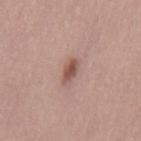Imaged during a routine full-body skin examination; the lesion was not biopsied and no histopathology is available.
A male subject approximately 30 years of age.
A close-up tile cropped from a whole-body skin photograph, about 15 mm across.
The tile uses white-light illumination.
The recorded lesion diameter is about 3 mm.
The total-body-photography lesion software estimated an area of roughly 3.5 mm², a shape eccentricity near 0.85, and two-axis asymmetry of about 0.2. The software also gave an average lesion color of about L≈52 a*≈21 b*≈24 (CIELAB), roughly 12 lightness units darker than nearby skin, and a normalized lesion–skin contrast near 8.5. And it measured internal color variation of about 2.5 on a 0–10 scale and a peripheral color-asymmetry measure near 1.